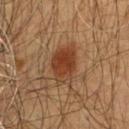Captured during whole-body skin photography for melanoma surveillance; the lesion was not biopsied.
A male subject aged 58 to 62.
A 15 mm crop from a total-body photograph taken for skin-cancer surveillance.
Imaged with cross-polarized lighting.
Measured at roughly 5 mm in maximum diameter.
The lesion is located on the chest.
Automated tile analysis of the lesion measured a lesion color around L≈37 a*≈21 b*≈31 in CIELAB, roughly 10 lightness units darker than nearby skin, and a normalized lesion–skin contrast near 8.5. And it measured a border-irregularity index near 2.5/10, a color-variation rating of about 5/10, and peripheral color asymmetry of about 1.5. And it measured a classifier nevus-likeness of about 95/100 and a detector confidence of about 100 out of 100 that the crop contains a lesion.